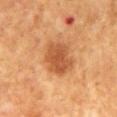Assessment: The lesion was photographed on a routine skin check and not biopsied; there is no pathology result. Acquisition and patient details: The lesion is on the mid back. Approximately 4.5 mm at its widest. A roughly 15 mm field-of-view crop from a total-body skin photograph. The total-body-photography lesion software estimated a lesion area of about 12 mm², an outline eccentricity of about 0.55 (0 = round, 1 = elongated), and a shape-asymmetry score of about 0.2 (0 = symmetric). And it measured a lesion–skin lightness drop of about 11 and a normalized border contrast of about 8. The analysis additionally found a border-irregularity index near 2/10 and internal color variation of about 3.5 on a 0–10 scale. A male subject, approximately 70 years of age. Captured under cross-polarized illumination.